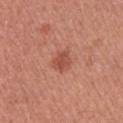workup=no biopsy performed (imaged during a skin exam); image source=total-body-photography crop, ~15 mm field of view; lighting=white-light illumination; patient=female, roughly 50 years of age; size=~2.5 mm (longest diameter); body site=the arm.